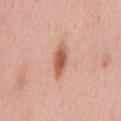notes = catalogued during a skin exam; not biopsied
body site = the mid back
patient = female, about 50 years old
tile lighting = white-light illumination
image source = 15 mm crop, total-body photography
diameter = ≈4 mm